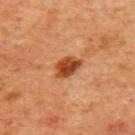workup — catalogued during a skin exam; not biopsied | imaging modality — ~15 mm tile from a whole-body skin photo | patient — male, aged 58–62 | tile lighting — cross-polarized | site — the front of the torso | lesion size — ≈3.5 mm.